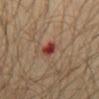follow-up: total-body-photography surveillance lesion; no biopsy
patient: male, roughly 30 years of age
automated lesion analysis: an area of roughly 3.5 mm², a shape eccentricity near 0.7, and a shape-asymmetry score of about 0.3 (0 = symmetric); a border-irregularity rating of about 2.5/10
lighting: cross-polarized
size: ~2.5 mm (longest diameter)
imaging modality: ~15 mm crop, total-body skin-cancer survey
body site: the abdomen